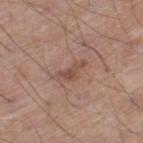Clinical impression:
The lesion was photographed on a routine skin check and not biopsied; there is no pathology result.
Context:
Automated image analysis of the tile measured roughly 9 lightness units darker than nearby skin and a lesion-to-skin contrast of about 7 (normalized; higher = more distinct). This is a white-light tile. Located on the left thigh. A male subject roughly 70 years of age. A lesion tile, about 15 mm wide, cut from a 3D total-body photograph. Measured at roughly 3.5 mm in maximum diameter.